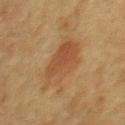The lesion was tiled from a total-body skin photograph and was not biopsied. The recorded lesion diameter is about 5.5 mm. A female subject in their 60s. Located on the upper back. Cropped from a total-body skin-imaging series; the visible field is about 15 mm.